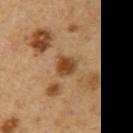Assessment:
The lesion was tiled from a total-body skin photograph and was not biopsied.
Clinical summary:
The lesion is on the right upper arm. The patient is a male about 55 years old. A lesion tile, about 15 mm wide, cut from a 3D total-body photograph.